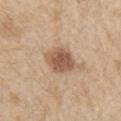Measured at roughly 3.5 mm in maximum diameter.
The lesion is located on the left upper arm.
A lesion tile, about 15 mm wide, cut from a 3D total-body photograph.
The tile uses white-light illumination.
A male subject aged approximately 70.
Automated tile analysis of the lesion measured a lesion area of about 9 mm², a shape eccentricity near 0.4, and a shape-asymmetry score of about 0.15 (0 = symmetric). The analysis additionally found a nevus-likeness score of about 90/100 and lesion-presence confidence of about 100/100.